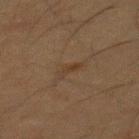Case summary:
– notes: catalogued during a skin exam; not biopsied
– subject: male, in their mid- to late 30s
– illumination: cross-polarized
– imaging modality: ~15 mm tile from a whole-body skin photo
– automated lesion analysis: a mean CIELAB color near L≈28 a*≈12 b*≈23, roughly 4 lightness units darker than nearby skin, and a normalized border contrast of about 5.5; internal color variation of about 0 on a 0–10 scale and peripheral color asymmetry of about 0; a nevus-likeness score of about 10/100
– site: the back